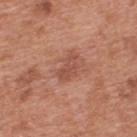Notes:
– notes — catalogued during a skin exam; not biopsied
– image source — ~15 mm crop, total-body skin-cancer survey
– illumination — white-light
– body site — the upper back
– patient — male, approximately 70 years of age
– size — about 3 mm